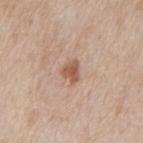No biopsy was performed on this lesion — it was imaged during a full skin examination and was not determined to be concerning. From the mid back. A 15 mm crop from a total-body photograph taken for skin-cancer surveillance. A male subject, in their mid- to late 60s. The recorded lesion diameter is about 3 mm.